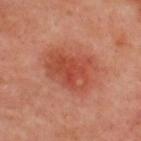Impression:
Captured during whole-body skin photography for melanoma surveillance; the lesion was not biopsied.
Image and clinical context:
From the back. A region of skin cropped from a whole-body photographic capture, roughly 15 mm wide. Measured at roughly 6 mm in maximum diameter. The patient is a male roughly 50 years of age.